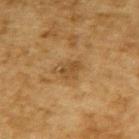Assessment:
The lesion was tiled from a total-body skin photograph and was not biopsied.
Context:
An algorithmic analysis of the crop reported an area of roughly 5.5 mm², a shape eccentricity near 0.7, and a shape-asymmetry score of about 0.35 (0 = symmetric). The software also gave a mean CIELAB color near L≈42 a*≈15 b*≈35, a lesion–skin lightness drop of about 7, and a normalized border contrast of about 6. The software also gave a border-irregularity rating of about 3.5/10 and internal color variation of about 2.5 on a 0–10 scale. It also reported a nevus-likeness score of about 0/100 and a detector confidence of about 100 out of 100 that the crop contains a lesion. The lesion's longest dimension is about 3.5 mm. This is a cross-polarized tile. A 15 mm crop from a total-body photograph taken for skin-cancer surveillance. A male patient aged 83 or over. On the back.